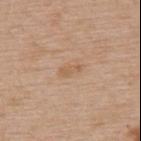Assessment: Recorded during total-body skin imaging; not selected for excision or biopsy. Context: Cropped from a whole-body photographic skin survey; the tile spans about 15 mm. The lesion is on the back. About 3 mm across. A female patient, aged around 65. This is a white-light tile.